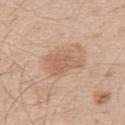lighting: white-light illumination; location: the upper back; subject: male, in their mid-50s; lesion size: ≈4 mm; image source: total-body-photography crop, ~15 mm field of view.